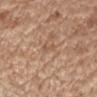Clinical impression:
This lesion was catalogued during total-body skin photography and was not selected for biopsy.
Clinical summary:
This image is a 15 mm lesion crop taken from a total-body photograph. From the right upper arm. Imaged with white-light lighting. A male patient aged 68–72. Measured at roughly 2.5 mm in maximum diameter. The lesion-visualizer software estimated a border-irregularity index near 4/10 and peripheral color asymmetry of about 0. And it measured lesion-presence confidence of about 50/100.